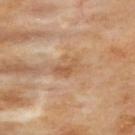biopsy status — imaged on a skin check; not biopsied
lighting — cross-polarized
size — ~3.5 mm (longest diameter)
image source — ~15 mm crop, total-body skin-cancer survey
body site — the upper back